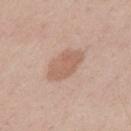Context:
A male patient, in their 40s. An algorithmic analysis of the crop reported an area of roughly 10 mm² and an eccentricity of roughly 0.8. The lesion's longest dimension is about 5 mm. A 15 mm close-up tile from a total-body photography series done for melanoma screening. From the mid back.